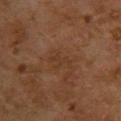Background: The total-body-photography lesion software estimated an outline eccentricity of about 0.9 (0 = round, 1 = elongated). And it measured an average lesion color of about L≈30 a*≈16 b*≈27 (CIELAB), a lesion–skin lightness drop of about 4, and a normalized border contrast of about 4.5. It also reported internal color variation of about 0 on a 0–10 scale. The patient is a female aged 58 to 62. The recorded lesion diameter is about 3 mm. A 15 mm crop from a total-body photograph taken for skin-cancer surveillance. The lesion is located on the upper back. Captured under cross-polarized illumination.